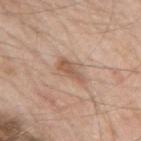| feature | finding |
|---|---|
| workup | total-body-photography surveillance lesion; no biopsy |
| tile lighting | white-light |
| image | ~15 mm crop, total-body skin-cancer survey |
| lesion size | ≈3.5 mm |
| site | the right upper arm |
| automated lesion analysis | a lesion color around L≈57 a*≈18 b*≈30 in CIELAB and a lesion-to-skin contrast of about 7 (normalized; higher = more distinct) |
| patient | male, about 60 years old |